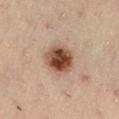Recorded during total-body skin imaging; not selected for excision or biopsy. The patient is a male aged approximately 55. A 15 mm crop from a total-body photograph taken for skin-cancer surveillance. Captured under cross-polarized illumination. About 4 mm across. From the left thigh.